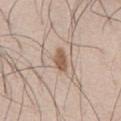Impression: Imaged during a routine full-body skin examination; the lesion was not biopsied and no histopathology is available. Image and clinical context: A male patient in their 60s. An algorithmic analysis of the crop reported an average lesion color of about L≈56 a*≈17 b*≈29 (CIELAB) and a lesion-to-skin contrast of about 8.5 (normalized; higher = more distinct). It also reported border irregularity of about 2 on a 0–10 scale, a color-variation rating of about 2/10, and peripheral color asymmetry of about 0.5. A 15 mm crop from a total-body photograph taken for skin-cancer surveillance. On the front of the torso. Measured at roughly 3 mm in maximum diameter. Captured under white-light illumination.